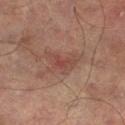The lesion is located on the left lower leg. The lesion's longest dimension is about 3.5 mm. The lesion-visualizer software estimated border irregularity of about 4.5 on a 0–10 scale, a within-lesion color-variation index near 3/10, and radial color variation of about 1. It also reported an automated nevus-likeness rating near 20 out of 100 and lesion-presence confidence of about 100/100. A male patient, aged 73–77. This is a cross-polarized tile. This image is a 15 mm lesion crop taken from a total-body photograph.